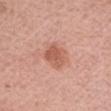Recorded during total-body skin imaging; not selected for excision or biopsy.
A female subject aged 63 to 67.
Approximately 4 mm at its widest.
This is a white-light tile.
The lesion is on the left forearm.
A 15 mm close-up tile from a total-body photography series done for melanoma screening.